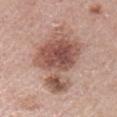subject: female, approximately 50 years of age | body site: the left upper arm | imaging modality: 15 mm crop, total-body photography | diameter: ≈10 mm | image-analysis metrics: an automated nevus-likeness rating near 75 out of 100 and a detector confidence of about 100 out of 100 that the crop contains a lesion | illumination: white-light.